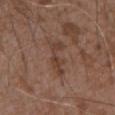The lesion was tiled from a total-body skin photograph and was not biopsied.